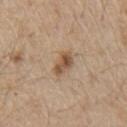Q: Was a biopsy performed?
A: catalogued during a skin exam; not biopsied
Q: How large is the lesion?
A: ~3 mm (longest diameter)
Q: Illumination type?
A: white-light illumination
Q: Patient demographics?
A: male, about 70 years old
Q: Where on the body is the lesion?
A: the front of the torso
Q: What kind of image is this?
A: ~15 mm tile from a whole-body skin photo